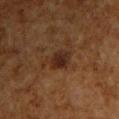Imaged during a routine full-body skin examination; the lesion was not biopsied and no histopathology is available.
About 3 mm across.
A close-up tile cropped from a whole-body skin photograph, about 15 mm across.
A male subject, roughly 60 years of age.
This is a cross-polarized tile.
The lesion is on the left upper arm.